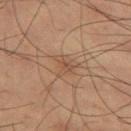This lesion was catalogued during total-body skin photography and was not selected for biopsy.
Imaged with cross-polarized lighting.
Measured at roughly 3 mm in maximum diameter.
A 15 mm close-up tile from a total-body photography series done for melanoma screening.
On the leg.
The patient is a male in their 60s.